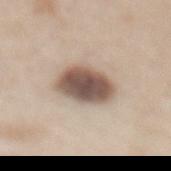  biopsy_status: not biopsied; imaged during a skin examination
  patient:
    sex: female
    age_approx: 50
  site: mid back
  automated_metrics:
    area_mm2_approx: 15.0
    eccentricity: 0.65
    cielab_L: 54
    cielab_a: 15
    cielab_b: 24
    vs_skin_darker_L: 19.0
    vs_skin_contrast_norm: 12.0
  image:
    source: total-body photography crop
    field_of_view_mm: 15
  lesion_size:
    long_diameter_mm_approx: 5.0